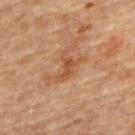notes: total-body-photography surveillance lesion; no biopsy | subject: male, aged 83 or over | lesion size: about 3 mm | site: the upper back | image source: 15 mm crop, total-body photography.